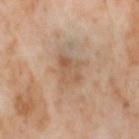Q: Was a biopsy performed?
A: imaged on a skin check; not biopsied
Q: What is the lesion's diameter?
A: ~3.5 mm (longest diameter)
Q: Patient demographics?
A: female, about 55 years old
Q: What did automated image analysis measure?
A: an outline eccentricity of about 0.6 (0 = round, 1 = elongated) and two-axis asymmetry of about 0.3; border irregularity of about 3 on a 0–10 scale and radial color variation of about 1.5
Q: Where on the body is the lesion?
A: the right thigh
Q: What is the imaging modality?
A: ~15 mm crop, total-body skin-cancer survey
Q: What lighting was used for the tile?
A: cross-polarized